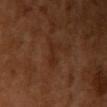Imaged during a routine full-body skin examination; the lesion was not biopsied and no histopathology is available.
The subject is a female about 55 years old.
A 15 mm close-up tile from a total-body photography series done for melanoma screening.
The lesion is located on the arm.